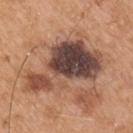Clinical impression:
The lesion was photographed on a routine skin check and not biopsied; there is no pathology result.
Image and clinical context:
The patient is a male in their mid- to late 50s. Automated image analysis of the tile measured a border-irregularity rating of about 6.5/10. And it measured an automated nevus-likeness rating near 0 out of 100 and a detector confidence of about 100 out of 100 that the crop contains a lesion. Located on the right upper arm. Imaged with white-light lighting. This image is a 15 mm lesion crop taken from a total-body photograph.